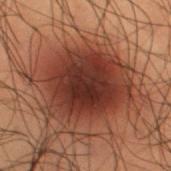Part of a total-body skin-imaging series; this lesion was reviewed on a skin check and was not flagged for biopsy. A region of skin cropped from a whole-body photographic capture, roughly 15 mm wide. Located on the right thigh. A male patient in their 50s. The lesion-visualizer software estimated a lesion area of about 44 mm², an outline eccentricity of about 0.6 (0 = round, 1 = elongated), and a symmetry-axis asymmetry near 0.2. The recorded lesion diameter is about 8.5 mm.